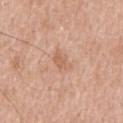notes: no biopsy performed (imaged during a skin exam); subject: male, aged approximately 60; body site: the front of the torso; image source: ~15 mm crop, total-body skin-cancer survey; illumination: white-light.